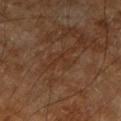Part of a total-body skin-imaging series; this lesion was reviewed on a skin check and was not flagged for biopsy. This is a cross-polarized tile. On the right forearm. The subject is a male approximately 85 years of age or older. An algorithmic analysis of the crop reported a lesion area of about 2.5 mm², a shape eccentricity near 0.75, and a shape-asymmetry score of about 0.8 (0 = symmetric). The analysis additionally found a mean CIELAB color near L≈31 a*≈18 b*≈29, roughly 4 lightness units darker than nearby skin, and a normalized lesion–skin contrast near 4.5. The software also gave border irregularity of about 9 on a 0–10 scale, a color-variation rating of about 0/10, and peripheral color asymmetry of about 0. A 15 mm close-up extracted from a 3D total-body photography capture. Measured at roughly 2.5 mm in maximum diameter.